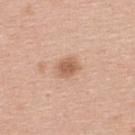The lesion was photographed on a routine skin check and not biopsied; there is no pathology result. Imaged with white-light lighting. Located on the upper back. A 15 mm close-up extracted from a 3D total-body photography capture. The total-body-photography lesion software estimated a mean CIELAB color near L≈60 a*≈21 b*≈32, about 11 CIELAB-L* units darker than the surrounding skin, and a normalized lesion–skin contrast near 7.5. The analysis additionally found a border-irregularity index near 1/10, internal color variation of about 2 on a 0–10 scale, and peripheral color asymmetry of about 0.5. It also reported a nevus-likeness score of about 80/100 and lesion-presence confidence of about 100/100. The lesion's longest dimension is about 3 mm. The subject is a female about 40 years old.